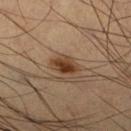Acquisition and patient details: An algorithmic analysis of the crop reported a footprint of about 5 mm² and a shape eccentricity near 0.75. And it measured a color-variation rating of about 4.5/10 and peripheral color asymmetry of about 2. It also reported a classifier nevus-likeness of about 95/100. The tile uses cross-polarized illumination. This image is a 15 mm lesion crop taken from a total-body photograph. A male subject in their mid-60s. The lesion is on the right lower leg.